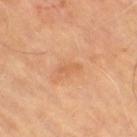<lesion>
<biopsy_status>not biopsied; imaged during a skin examination</biopsy_status>
<lighting>cross-polarized</lighting>
<image>
  <source>total-body photography crop</source>
  <field_of_view_mm>15</field_of_view_mm>
</image>
<patient>
  <age_approx>65</age_approx>
</patient>
<automated_metrics>
  <border_irregularity_0_10>4.5</border_irregularity_0_10>
  <peripheral_color_asymmetry>0.0</peripheral_color_asymmetry>
  <nevus_likeness_0_100>0</nevus_likeness_0_100>
</automated_metrics>
<site>right thigh</site>
</lesion>